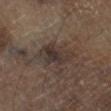Captured during whole-body skin photography for melanoma surveillance; the lesion was not biopsied.
Captured under cross-polarized illumination.
The patient is a male in their mid-60s.
A 15 mm close-up tile from a total-body photography series done for melanoma screening.
The lesion is located on the left lower leg.
Automated image analysis of the tile measured a lesion color around L≈31 a*≈11 b*≈17 in CIELAB, about 7 CIELAB-L* units darker than the surrounding skin, and a normalized lesion–skin contrast near 8. And it measured a border-irregularity rating of about 3/10 and peripheral color asymmetry of about 1.5.